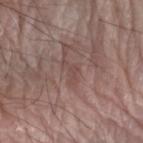Assessment: This lesion was catalogued during total-body skin photography and was not selected for biopsy. Acquisition and patient details: The tile uses white-light illumination. The patient is a male approximately 80 years of age. Cropped from a total-body skin-imaging series; the visible field is about 15 mm. Measured at roughly 3 mm in maximum diameter. Located on the right arm.